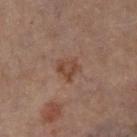The lesion's longest dimension is about 3 mm. The lesion is on the leg. A 15 mm close-up extracted from a 3D total-body photography capture. The subject is a female approximately 60 years of age. Automated tile analysis of the lesion measured a mean CIELAB color near L≈44 a*≈19 b*≈27 and roughly 7 lightness units darker than nearby skin. And it measured a border-irregularity rating of about 3.5/10 and a peripheral color-asymmetry measure near 1.